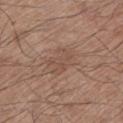No biopsy was performed on this lesion — it was imaged during a full skin examination and was not determined to be concerning. Imaged with white-light lighting. The patient is a male approximately 65 years of age. The recorded lesion diameter is about 4 mm. Automated tile analysis of the lesion measured a footprint of about 9 mm², an eccentricity of roughly 0.7, and two-axis asymmetry of about 0.25. The software also gave a lesion-to-skin contrast of about 4.5 (normalized; higher = more distinct). The analysis additionally found a classifier nevus-likeness of about 0/100 and a detector confidence of about 100 out of 100 that the crop contains a lesion. Cropped from a whole-body photographic skin survey; the tile spans about 15 mm. On the left lower leg.